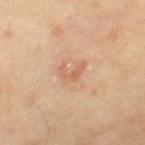This lesion was catalogued during total-body skin photography and was not selected for biopsy. Longest diameter approximately 3 mm. Imaged with cross-polarized lighting. A roughly 15 mm field-of-view crop from a total-body skin photograph. The patient is a male aged 63–67. On the right thigh.